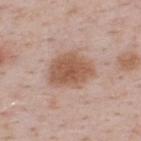Imaged during a routine full-body skin examination; the lesion was not biopsied and no histopathology is available.
Longest diameter approximately 5 mm.
A 15 mm close-up tile from a total-body photography series done for melanoma screening.
Imaged with white-light lighting.
Located on the upper back.
Automated image analysis of the tile measured a footprint of about 15 mm² and a shape eccentricity near 0.65. It also reported an average lesion color of about L≈55 a*≈20 b*≈29 (CIELAB), about 11 CIELAB-L* units darker than the surrounding skin, and a lesion-to-skin contrast of about 8.5 (normalized; higher = more distinct). And it measured a border-irregularity rating of about 2.5/10, a color-variation rating of about 2.5/10, and peripheral color asymmetry of about 0.5. The analysis additionally found an automated nevus-likeness rating near 90 out of 100 and lesion-presence confidence of about 100/100.
A male subject, aged 48–52.